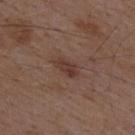biopsy_status: not biopsied; imaged during a skin examination
patient:
  sex: male
  age_approx: 50
site: mid back
image:
  source: total-body photography crop
  field_of_view_mm: 15